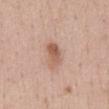biopsy status: catalogued during a skin exam; not biopsied | site: the abdomen | patient: female, aged 43–47 | lesion diameter: about 3.5 mm | image-analysis metrics: internal color variation of about 5 on a 0–10 scale and peripheral color asymmetry of about 2 | image source: total-body-photography crop, ~15 mm field of view | lighting: white-light.